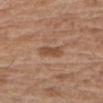– workup — imaged on a skin check; not biopsied
– patient — male, aged 68 to 72
– lesion size — about 3 mm
– image — ~15 mm tile from a whole-body skin photo
– location — the right forearm
– tile lighting — white-light